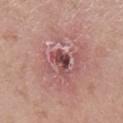Imaged during a routine full-body skin examination; the lesion was not biopsied and no histopathology is available.
The patient is a female aged approximately 70.
Measured at roughly 3 mm in maximum diameter.
This image is a 15 mm lesion crop taken from a total-body photograph.
The lesion is located on the left lower leg.
Captured under white-light illumination.